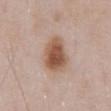  biopsy_status: not biopsied; imaged during a skin examination
  automated_metrics:
    area_mm2_approx: 13.0
    eccentricity: 0.7
  image:
    source: total-body photography crop
    field_of_view_mm: 15
  site: abdomen
  lesion_size:
    long_diameter_mm_approx: 4.5
  patient:
    sex: male
    age_approx: 55